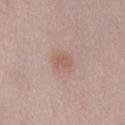notes: catalogued during a skin exam; not biopsied | body site: the lower back | patient: female, approximately 50 years of age | image: 15 mm crop, total-body photography.